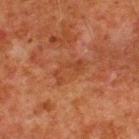Clinical impression: This lesion was catalogued during total-body skin photography and was not selected for biopsy. Background: A roughly 15 mm field-of-view crop from a total-body skin photograph. The tile uses cross-polarized illumination. The subject is a male about 80 years old. Automated image analysis of the tile measured a lesion area of about 4.5 mm², an eccentricity of roughly 0.9, and a symmetry-axis asymmetry near 0.6. And it measured an average lesion color of about L≈34 a*≈23 b*≈30 (CIELAB), roughly 5 lightness units darker than nearby skin, and a normalized lesion–skin contrast near 5. On the upper back.